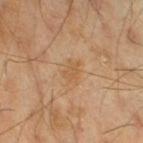<tbp_lesion>
<biopsy_status>not biopsied; imaged during a skin examination</biopsy_status>
<lesion_size>
  <long_diameter_mm_approx>2.5</long_diameter_mm_approx>
</lesion_size>
<image>
  <source>total-body photography crop</source>
  <field_of_view_mm>15</field_of_view_mm>
</image>
<site>leg</site>
<lighting>cross-polarized</lighting>
<patient>
  <sex>male</sex>
  <age_approx>70</age_approx>
</patient>
</tbp_lesion>